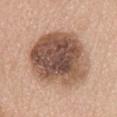Impression:
This lesion was catalogued during total-body skin photography and was not selected for biopsy.
Acquisition and patient details:
A female patient aged approximately 60. Approximately 7.5 mm at its widest. A region of skin cropped from a whole-body photographic capture, roughly 15 mm wide. The total-body-photography lesion software estimated a lesion area of about 43 mm², an outline eccentricity of about 0.35 (0 = round, 1 = elongated), and a shape-asymmetry score of about 0.15 (0 = symmetric). The software also gave a mean CIELAB color near L≈51 a*≈19 b*≈27, about 18 CIELAB-L* units darker than the surrounding skin, and a lesion-to-skin contrast of about 11.5 (normalized; higher = more distinct). On the abdomen.